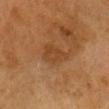| key | value |
|---|---|
| biopsy status | catalogued during a skin exam; not biopsied |
| diameter | ~3.5 mm (longest diameter) |
| illumination | cross-polarized illumination |
| anatomic site | the head or neck |
| patient | female, aged 63–67 |
| image | ~15 mm crop, total-body skin-cancer survey |